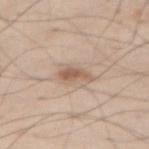Context: The lesion-visualizer software estimated a footprint of about 4.5 mm², an eccentricity of roughly 0.85, and two-axis asymmetry of about 0.35. The analysis additionally found an average lesion color of about L≈58 a*≈17 b*≈28 (CIELAB) and a lesion-to-skin contrast of about 7.5 (normalized; higher = more distinct). It also reported border irregularity of about 3.5 on a 0–10 scale, internal color variation of about 2.5 on a 0–10 scale, and a peripheral color-asymmetry measure near 1. The analysis additionally found a classifier nevus-likeness of about 60/100 and lesion-presence confidence of about 100/100. Longest diameter approximately 3.5 mm. A region of skin cropped from a whole-body photographic capture, roughly 15 mm wide. From the front of the torso. A male patient aged around 60.